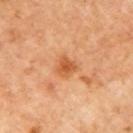Notes:
* follow-up: imaged on a skin check; not biopsied
* image: 15 mm crop, total-body photography
* subject: female, about 50 years old
* illumination: cross-polarized
* anatomic site: the upper back